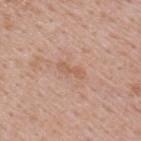Q: Was a biopsy performed?
A: imaged on a skin check; not biopsied
Q: Who is the patient?
A: male, in their 40s
Q: Where on the body is the lesion?
A: the upper back
Q: How was this image acquired?
A: total-body-photography crop, ~15 mm field of view